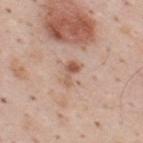Captured during whole-body skin photography for melanoma surveillance; the lesion was not biopsied. This is a white-light tile. Located on the upper back. This image is a 15 mm lesion crop taken from a total-body photograph. A male patient, roughly 35 years of age.